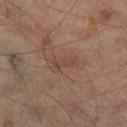biopsy_status: not biopsied; imaged during a skin examination
automated_metrics:
  border_irregularity_0_10: 5.5
  color_variation_0_10: 0.0
  peripheral_color_asymmetry: 0.0
  nevus_likeness_0_100: 0
  lesion_detection_confidence_0_100: 100
site: right lower leg
patient:
  sex: male
  age_approx: 65
image:
  source: total-body photography crop
  field_of_view_mm: 15
lesion_size:
  long_diameter_mm_approx: 3.5
lighting: cross-polarized